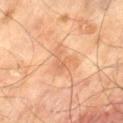{"biopsy_status": "not biopsied; imaged during a skin examination", "patient": {"sex": "male", "age_approx": 70}, "image": {"source": "total-body photography crop", "field_of_view_mm": 15}, "site": "left thigh"}